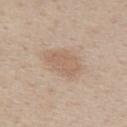Clinical impression:
The lesion was photographed on a routine skin check and not biopsied; there is no pathology result.
Clinical summary:
A female patient, about 45 years old. About 5.5 mm across. From the back. This is a white-light tile. The total-body-photography lesion software estimated a lesion area of about 15 mm², a shape eccentricity near 0.75, and a shape-asymmetry score of about 0.2 (0 = symmetric). The analysis additionally found an average lesion color of about L≈62 a*≈15 b*≈29 (CIELAB), about 7 CIELAB-L* units darker than the surrounding skin, and a lesion-to-skin contrast of about 5 (normalized; higher = more distinct). The software also gave border irregularity of about 2.5 on a 0–10 scale, a within-lesion color-variation index near 2/10, and peripheral color asymmetry of about 0.5. A 15 mm close-up extracted from a 3D total-body photography capture.